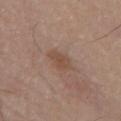This lesion was catalogued during total-body skin photography and was not selected for biopsy. Longest diameter approximately 3 mm. A male subject aged around 80. Cropped from a total-body skin-imaging series; the visible field is about 15 mm. On the back. Captured under white-light illumination.